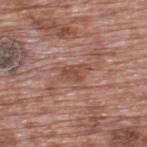Impression: The lesion was photographed on a routine skin check and not biopsied; there is no pathology result. Background: Longest diameter approximately 3.5 mm. A male subject approximately 70 years of age. The lesion is on the upper back. A lesion tile, about 15 mm wide, cut from a 3D total-body photograph.